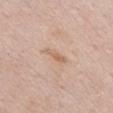follow-up=catalogued during a skin exam; not biopsied
subject=female, aged approximately 70
TBP lesion metrics=a lesion area of about 3 mm² and an eccentricity of roughly 0.95; an average lesion color of about L≈63 a*≈18 b*≈32 (CIELAB)
acquisition=total-body-photography crop, ~15 mm field of view
anatomic site=the front of the torso
lighting=white-light
size=about 3 mm